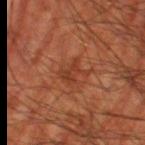Recorded during total-body skin imaging; not selected for excision or biopsy. Cropped from a total-body skin-imaging series; the visible field is about 15 mm. Measured at roughly 3 mm in maximum diameter. On the right thigh. A male patient aged 58 to 62. Imaged with cross-polarized lighting.